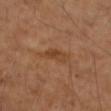Impression:
The lesion was tiled from a total-body skin photograph and was not biopsied.
Clinical summary:
A lesion tile, about 15 mm wide, cut from a 3D total-body photograph. From the right upper arm. This is a cross-polarized tile. A female patient about 60 years old. The total-body-photography lesion software estimated border irregularity of about 5 on a 0–10 scale, a within-lesion color-variation index near 3.5/10, and radial color variation of about 1. It also reported a nevus-likeness score of about 5/100.